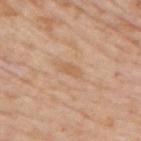Q: Is there a histopathology result?
A: catalogued during a skin exam; not biopsied
Q: What lighting was used for the tile?
A: white-light
Q: How was this image acquired?
A: ~15 mm tile from a whole-body skin photo
Q: Where on the body is the lesion?
A: the upper back
Q: What are the patient's age and sex?
A: male, aged 73–77